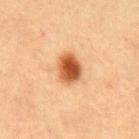<tbp_lesion>
  <patient>
    <sex>female</sex>
    <age_approx>55</age_approx>
  </patient>
  <site>front of the torso</site>
  <automated_metrics>
    <cielab_L>54</cielab_L>
    <cielab_a>26</cielab_a>
    <cielab_b>40</cielab_b>
    <vs_skin_darker_L>18.0</vs_skin_darker_L>
    <vs_skin_contrast_norm>11.5</vs_skin_contrast_norm>
    <nevus_likeness_0_100>100</nevus_likeness_0_100>
    <lesion_detection_confidence_0_100>100</lesion_detection_confidence_0_100>
  </automated_metrics>
  <image>
    <source>total-body photography crop</source>
    <field_of_view_mm>15</field_of_view_mm>
  </image>
  <lighting>cross-polarized</lighting>
</tbp_lesion>